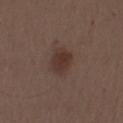biopsy status — imaged on a skin check; not biopsied | size — about 3.5 mm | acquisition — ~15 mm crop, total-body skin-cancer survey | tile lighting — white-light | TBP lesion metrics — a lesion–skin lightness drop of about 8 and a lesion-to-skin contrast of about 7.5 (normalized; higher = more distinct); a border-irregularity rating of about 2/10, internal color variation of about 3 on a 0–10 scale, and a peripheral color-asymmetry measure near 1; an automated nevus-likeness rating near 90 out of 100 and a lesion-detection confidence of about 100/100 | patient — male, about 70 years old | body site — the left upper arm.